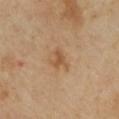Case summary:
• notes: total-body-photography surveillance lesion; no biopsy
• patient: female, about 40 years old
• acquisition: ~15 mm tile from a whole-body skin photo
• lighting: cross-polarized illumination
• lesion diameter: ~2.5 mm (longest diameter)
• anatomic site: the chest